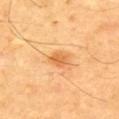  biopsy_status: not biopsied; imaged during a skin examination
  automated_metrics:
    cielab_L: 65
    cielab_a: 27
    cielab_b: 47
    vs_skin_darker_L: 10.0
    vs_skin_contrast_norm: 7.0
    border_irregularity_0_10: 2.5
    color_variation_0_10: 2.5
    peripheral_color_asymmetry: 1.0
    nevus_likeness_0_100: 80
  lesion_size:
    long_diameter_mm_approx: 2.5
  lighting: cross-polarized
  patient:
    sex: male
    age_approx: 55
  site: upper back
  image:
    source: total-body photography crop
    field_of_view_mm: 15